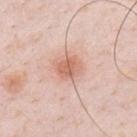Captured during whole-body skin photography for melanoma surveillance; the lesion was not biopsied. This is a white-light tile. The subject is a male aged approximately 35. About 3 mm across. From the chest. A 15 mm close-up extracted from a 3D total-body photography capture.